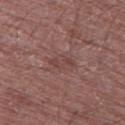The lesion is located on the leg. Automated tile analysis of the lesion measured a lesion area of about 3 mm², an outline eccentricity of about 0.9 (0 = round, 1 = elongated), and two-axis asymmetry of about 0.6. The analysis additionally found an average lesion color of about L≈42 a*≈21 b*≈22 (CIELAB) and a normalized border contrast of about 5.5. Approximately 3 mm at its widest. Cropped from a whole-body photographic skin survey; the tile spans about 15 mm. The subject is a male about 70 years old.